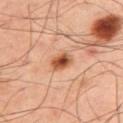Acquisition and patient details: The subject is a male about 50 years old. The recorded lesion diameter is about 3 mm. A region of skin cropped from a whole-body photographic capture, roughly 15 mm wide. This is a cross-polarized tile. Located on the upper back.